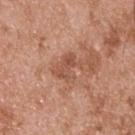Acquisition and patient details: The lesion is located on the back. The total-body-photography lesion software estimated an eccentricity of roughly 0.7. The software also gave a mean CIELAB color near L≈52 a*≈24 b*≈31, a lesion–skin lightness drop of about 8, and a normalized lesion–skin contrast near 6. It also reported border irregularity of about 5.5 on a 0–10 scale, a color-variation rating of about 4.5/10, and peripheral color asymmetry of about 1.5. It also reported a nevus-likeness score of about 0/100 and lesion-presence confidence of about 100/100. Cropped from a total-body skin-imaging series; the visible field is about 15 mm. A male patient, roughly 55 years of age.